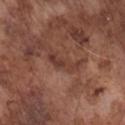Captured during whole-body skin photography for melanoma surveillance; the lesion was not biopsied.
The patient is a male roughly 75 years of age.
A lesion tile, about 15 mm wide, cut from a 3D total-body photograph.
The recorded lesion diameter is about 4.5 mm.
The tile uses white-light illumination.
From the chest.